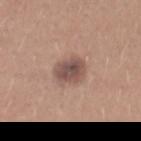The lesion was photographed on a routine skin check and not biopsied; there is no pathology result. From the abdomen. The patient is a female in their mid- to late 20s. A region of skin cropped from a whole-body photographic capture, roughly 15 mm wide.